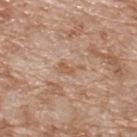The lesion was photographed on a routine skin check and not biopsied; there is no pathology result.
A close-up tile cropped from a whole-body skin photograph, about 15 mm across.
Automated tile analysis of the lesion measured a border-irregularity rating of about 5.5/10, a color-variation rating of about 0/10, and radial color variation of about 0. And it measured a nevus-likeness score of about 0/100 and lesion-presence confidence of about 95/100.
A male subject, aged 78 to 82.
This is a white-light tile.
Longest diameter approximately 3 mm.
On the upper back.